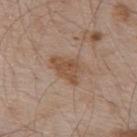Q: Was this lesion biopsied?
A: no biopsy performed (imaged during a skin exam)